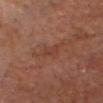image source = ~15 mm crop, total-body skin-cancer survey; body site = the head or neck; subject = male, roughly 60 years of age; tile lighting = cross-polarized; automated lesion analysis = a nevus-likeness score of about 0/100 and lesion-presence confidence of about 100/100; size = ~2.5 mm (longest diameter).